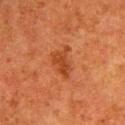biopsy_status: not biopsied; imaged during a skin examination
site: left upper arm
automated_metrics:
  area_mm2_approx: 6.5
  eccentricity: 0.7
  shape_asymmetry: 0.45
  cielab_L: 38
  cielab_a: 27
  cielab_b: 36
  vs_skin_darker_L: 8.0
  border_irregularity_0_10: 5.0
  color_variation_0_10: 1.5
  nevus_likeness_0_100: 40
  lesion_detection_confidence_0_100: 100
patient:
  sex: female
  age_approx: 50
image:
  source: total-body photography crop
  field_of_view_mm: 15
lighting: cross-polarized